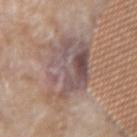<tbp_lesion>
  <biopsy_status>not biopsied; imaged during a skin examination</biopsy_status>
  <image>
    <source>total-body photography crop</source>
    <field_of_view_mm>15</field_of_view_mm>
  </image>
  <patient>
    <sex>male</sex>
    <age_approx>80</age_approx>
  </patient>
  <site>left forearm</site>
</tbp_lesion>